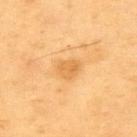Q: Was a biopsy performed?
A: total-body-photography surveillance lesion; no biopsy
Q: Automated lesion metrics?
A: a mean CIELAB color near L≈67 a*≈23 b*≈49 and about 9 CIELAB-L* units darker than the surrounding skin; a border-irregularity index near 2/10, a color-variation rating of about 2.5/10, and a peripheral color-asymmetry measure near 1; a nevus-likeness score of about 0/100 and a detector confidence of about 100 out of 100 that the crop contains a lesion
Q: Where on the body is the lesion?
A: the upper back
Q: What are the patient's age and sex?
A: male, aged 58 to 62
Q: Lesion size?
A: about 3 mm
Q: How was this image acquired?
A: ~15 mm tile from a whole-body skin photo
Q: Illumination type?
A: cross-polarized